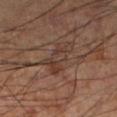Captured during whole-body skin photography for melanoma surveillance; the lesion was not biopsied.
A 15 mm crop from a total-body photograph taken for skin-cancer surveillance.
The lesion is located on the left lower leg.
A male patient, about 60 years old.